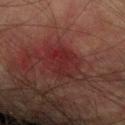{"biopsy_status": "not biopsied; imaged during a skin examination", "image": {"source": "total-body photography crop", "field_of_view_mm": 15}, "lighting": "cross-polarized", "automated_metrics": {"area_mm2_approx": 11.0, "shape_asymmetry": 0.2, "nevus_likeness_0_100": 0}, "lesion_size": {"long_diameter_mm_approx": 4.5}, "site": "right forearm", "patient": {"sex": "male", "age_approx": 75}}